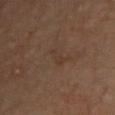{
  "biopsy_status": "not biopsied; imaged during a skin examination",
  "site": "chest",
  "lesion_size": {
    "long_diameter_mm_approx": 3.0
  },
  "patient": {
    "sex": "male",
    "age_approx": 65
  },
  "image": {
    "source": "total-body photography crop",
    "field_of_view_mm": 15
  }
}